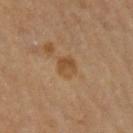No biopsy was performed on this lesion — it was imaged during a full skin examination and was not determined to be concerning. The lesion is located on the right upper arm. Measured at roughly 3 mm in maximum diameter. A roughly 15 mm field-of-view crop from a total-body skin photograph. The lesion-visualizer software estimated a mean CIELAB color near L≈45 a*≈17 b*≈33, a lesion–skin lightness drop of about 7, and a normalized lesion–skin contrast near 6.5. This is a cross-polarized tile. The patient is a female in their mid-60s.